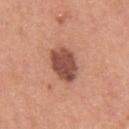Clinical impression:
Part of a total-body skin-imaging series; this lesion was reviewed on a skin check and was not flagged for biopsy.
Context:
A male patient, aged 28–32. About 4 mm across. Automated tile analysis of the lesion measured an area of roughly 11 mm², a shape eccentricity near 0.6, and two-axis asymmetry of about 0.1. And it measured about 15 CIELAB-L* units darker than the surrounding skin and a normalized border contrast of about 10. It also reported border irregularity of about 1.5 on a 0–10 scale, internal color variation of about 4 on a 0–10 scale, and a peripheral color-asymmetry measure near 1.5. The analysis additionally found a classifier nevus-likeness of about 35/100 and a lesion-detection confidence of about 100/100. The lesion is located on the upper back. This image is a 15 mm lesion crop taken from a total-body photograph. Captured under white-light illumination.